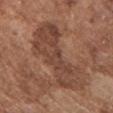Q: Is there a histopathology result?
A: total-body-photography surveillance lesion; no biopsy
Q: How was the tile lit?
A: white-light illumination
Q: What is the imaging modality?
A: 15 mm crop, total-body photography
Q: Lesion location?
A: the chest
Q: Patient demographics?
A: male, aged approximately 75
Q: Lesion size?
A: about 9 mm